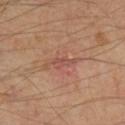Impression:
Captured during whole-body skin photography for melanoma surveillance; the lesion was not biopsied.
Acquisition and patient details:
Longest diameter approximately 2.5 mm. Imaged with cross-polarized lighting. From the right lower leg. A 15 mm close-up tile from a total-body photography series done for melanoma screening. Automated image analysis of the tile measured an area of roughly 3 mm², an outline eccentricity of about 0.9 (0 = round, 1 = elongated), and a shape-asymmetry score of about 0.35 (0 = symmetric). The software also gave an average lesion color of about L≈49 a*≈23 b*≈27 (CIELAB) and a normalized border contrast of about 5.5. A subject roughly 55 years of age.